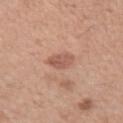Captured during whole-body skin photography for melanoma surveillance; the lesion was not biopsied. From the left upper arm. About 2.5 mm across. A male patient in their mid-40s. The tile uses white-light illumination. This image is a 15 mm lesion crop taken from a total-body photograph.